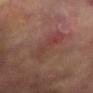Q: Is there a histopathology result?
A: catalogued during a skin exam; not biopsied
Q: What is the imaging modality?
A: ~15 mm crop, total-body skin-cancer survey
Q: Illumination type?
A: cross-polarized illumination
Q: What is the anatomic site?
A: the right arm
Q: Lesion size?
A: ~6 mm (longest diameter)
Q: What are the patient's age and sex?
A: female, aged 78 to 82